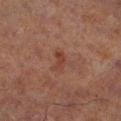Impression:
Part of a total-body skin-imaging series; this lesion was reviewed on a skin check and was not flagged for biopsy.
Clinical summary:
A male subject, in their 70s. The recorded lesion diameter is about 2.5 mm. This is a cross-polarized tile. From the left lower leg. Cropped from a total-body skin-imaging series; the visible field is about 15 mm. Automated tile analysis of the lesion measured an automated nevus-likeness rating near 0 out of 100.